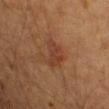Assessment: Part of a total-body skin-imaging series; this lesion was reviewed on a skin check and was not flagged for biopsy. Clinical summary: A male subject, roughly 55 years of age. This is a cross-polarized tile. An algorithmic analysis of the crop reported a mean CIELAB color near L≈36 a*≈21 b*≈29 and a normalized lesion–skin contrast near 6. And it measured radial color variation of about 1. A 15 mm close-up extracted from a 3D total-body photography capture. The lesion is on the left upper arm.